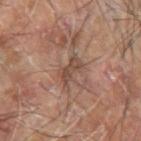Imaged during a routine full-body skin examination; the lesion was not biopsied and no histopathology is available. A male subject, aged 63 to 67. The tile uses cross-polarized illumination. From the arm. A roughly 15 mm field-of-view crop from a total-body skin photograph.